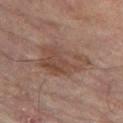Captured under cross-polarized illumination. A 15 mm close-up extracted from a 3D total-body photography capture. A female patient aged approximately 80. Measured at roughly 6 mm in maximum diameter. The lesion is located on the leg. The total-body-photography lesion software estimated an area of roughly 17 mm², a shape eccentricity near 0.7, and a shape-asymmetry score of about 0.35 (0 = symmetric). And it measured roughly 7 lightness units darker than nearby skin and a lesion-to-skin contrast of about 6.5 (normalized; higher = more distinct). It also reported a nevus-likeness score of about 5/100 and a detector confidence of about 100 out of 100 that the crop contains a lesion.